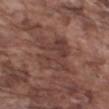Notes:
- follow-up: catalogued during a skin exam; not biopsied
- imaging modality: total-body-photography crop, ~15 mm field of view
- lesion diameter: about 6 mm
- subject: male, approximately 75 years of age
- illumination: white-light
- image-analysis metrics: a lesion–skin lightness drop of about 7 and a normalized border contrast of about 6; a within-lesion color-variation index near 4/10
- location: the right upper arm